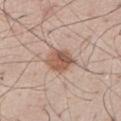notes=no biopsy performed (imaged during a skin exam)
automated lesion analysis=an eccentricity of roughly 0.45 and two-axis asymmetry of about 0.2; a mean CIELAB color near L≈55 a*≈19 b*≈28, a lesion–skin lightness drop of about 12, and a normalized lesion–skin contrast near 8.5; internal color variation of about 5.5 on a 0–10 scale and peripheral color asymmetry of about 2; a classifier nevus-likeness of about 90/100 and a lesion-detection confidence of about 100/100
subject=male, aged approximately 60
anatomic site=the chest
tile lighting=white-light
lesion diameter=~3 mm (longest diameter)
image source=total-body-photography crop, ~15 mm field of view